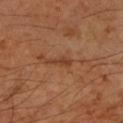Part of a total-body skin-imaging series; this lesion was reviewed on a skin check and was not flagged for biopsy.
An algorithmic analysis of the crop reported an area of roughly 2.5 mm², an outline eccentricity of about 0.95 (0 = round, 1 = elongated), and two-axis asymmetry of about 0.4.
Imaged with cross-polarized lighting.
The lesion is on the left forearm.
A region of skin cropped from a whole-body photographic capture, roughly 15 mm wide.
A male subject approximately 60 years of age.